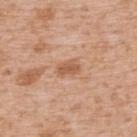Case summary:
- biopsy status · total-body-photography surveillance lesion; no biopsy
- patient · male, in their mid- to late 60s
- imaging modality · ~15 mm tile from a whole-body skin photo
- body site · the upper back
- image-analysis metrics · an average lesion color of about L≈57 a*≈22 b*≈34 (CIELAB), a lesion–skin lightness drop of about 10, and a normalized lesion–skin contrast near 7; an automated nevus-likeness rating near 10 out of 100 and a detector confidence of about 100 out of 100 that the crop contains a lesion
- tile lighting · white-light
- diameter · ≈2.5 mm